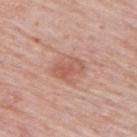biopsy_status: not biopsied; imaged during a skin examination
lesion_size:
  long_diameter_mm_approx: 3.5
site: upper back
image:
  source: total-body photography crop
  field_of_view_mm: 15
patient:
  sex: male
  age_approx: 65